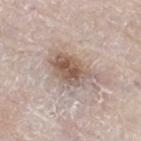Image and clinical context: Captured under white-light illumination. Longest diameter approximately 6 mm. A male patient, approximately 80 years of age. On the right thigh. A close-up tile cropped from a whole-body skin photograph, about 15 mm across.